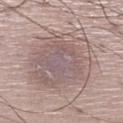Q: Is there a histopathology result?
A: imaged on a skin check; not biopsied
Q: Where on the body is the lesion?
A: the leg
Q: What kind of image is this?
A: ~15 mm crop, total-body skin-cancer survey
Q: What lighting was used for the tile?
A: white-light
Q: What is the lesion's diameter?
A: ~8.5 mm (longest diameter)
Q: What are the patient's age and sex?
A: male, in their 50s